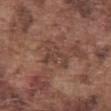Captured during whole-body skin photography for melanoma surveillance; the lesion was not biopsied. A male patient, aged 73 to 77. Captured under white-light illumination. Longest diameter approximately 5 mm. The lesion is on the front of the torso. A close-up tile cropped from a whole-body skin photograph, about 15 mm across.